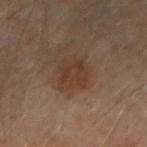Q: Is there a histopathology result?
A: total-body-photography surveillance lesion; no biopsy
Q: What are the patient's age and sex?
A: aged approximately 55
Q: Where on the body is the lesion?
A: the right forearm
Q: Automated lesion metrics?
A: a lesion area of about 10 mm², an outline eccentricity of about 0.45 (0 = round, 1 = elongated), and two-axis asymmetry of about 0.2
Q: How was this image acquired?
A: ~15 mm tile from a whole-body skin photo